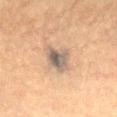{
  "automated_metrics": {
    "area_mm2_approx": 7.5,
    "eccentricity": 0.65,
    "shape_asymmetry": 0.25,
    "cielab_L": 50,
    "cielab_a": 11,
    "cielab_b": 22,
    "vs_skin_darker_L": 11.0,
    "vs_skin_contrast_norm": 10.0,
    "border_irregularity_0_10": 2.5,
    "color_variation_0_10": 7.0,
    "nevus_likeness_0_100": 80
  },
  "lesion_size": {
    "long_diameter_mm_approx": 4.0
  },
  "site": "chest",
  "image": {
    "source": "total-body photography crop",
    "field_of_view_mm": 15
  },
  "lighting": "cross-polarized",
  "patient": {
    "sex": "female",
    "age_approx": 70
  }
}